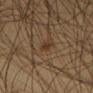The lesion was tiled from a total-body skin photograph and was not biopsied. Imaged with cross-polarized lighting. The subject is a male roughly 45 years of age. Approximately 1.5 mm at its widest. The lesion is on the left thigh. The total-body-photography lesion software estimated a footprint of about 1.5 mm², a shape eccentricity near 0.8, and a symmetry-axis asymmetry near 0.4. It also reported a lesion–skin lightness drop of about 7 and a normalized lesion–skin contrast near 7. It also reported a border-irregularity rating of about 3/10 and a color-variation rating of about 0/10. This image is a 15 mm lesion crop taken from a total-body photograph.